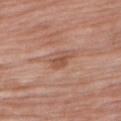Recorded during total-body skin imaging; not selected for excision or biopsy. This image is a 15 mm lesion crop taken from a total-body photograph. The lesion is located on the right upper arm. A female patient in their 70s.